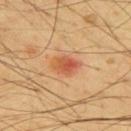Located on the upper back.
The patient is a male aged approximately 65.
A 15 mm crop from a total-body photograph taken for skin-cancer surveillance.